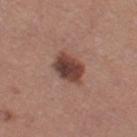follow-up=total-body-photography surveillance lesion; no biopsy
patient=female, aged 33 to 37
body site=the left thigh
size=about 4 mm
acquisition=~15 mm tile from a whole-body skin photo
lighting=white-light illumination
automated lesion analysis=border irregularity of about 2.5 on a 0–10 scale, internal color variation of about 5.5 on a 0–10 scale, and a peripheral color-asymmetry measure near 1.5